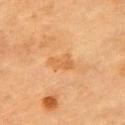biopsy status: total-body-photography surveillance lesion; no biopsy | image-analysis metrics: a shape eccentricity near 0.85 and a shape-asymmetry score of about 0.35 (0 = symmetric); a border-irregularity index near 3.5/10, a within-lesion color-variation index near 2.5/10, and radial color variation of about 1; an automated nevus-likeness rating near 0 out of 100 | diameter: ~3.5 mm (longest diameter) | patient: male, aged 83 or older | location: the chest | image: ~15 mm crop, total-body skin-cancer survey | tile lighting: cross-polarized.